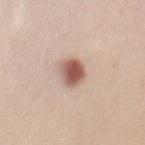notes: total-body-photography surveillance lesion; no biopsy.